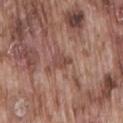Acquisition and patient details:
A close-up tile cropped from a whole-body skin photograph, about 15 mm across. A male subject aged around 75. Located on the lower back.